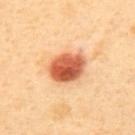Case summary:
• notes: no biopsy performed (imaged during a skin exam)
• subject: male, aged around 40
• site: the upper back
• tile lighting: cross-polarized
• lesion size: ~4.5 mm (longest diameter)
• acquisition: ~15 mm tile from a whole-body skin photo